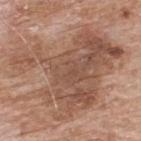This lesion was catalogued during total-body skin photography and was not selected for biopsy. A male subject aged approximately 65. Imaged with white-light lighting. Automated tile analysis of the lesion measured a color-variation rating of about 6/10. The analysis additionally found an automated nevus-likeness rating near 0 out of 100 and a lesion-detection confidence of about 95/100. On the upper back. A close-up tile cropped from a whole-body skin photograph, about 15 mm across.